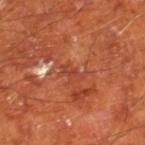biopsy status — total-body-photography surveillance lesion; no biopsy | patient — male, aged around 65 | lighting — cross-polarized illumination | site — the leg | image source — total-body-photography crop, ~15 mm field of view.